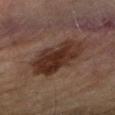Part of a total-body skin-imaging series; this lesion was reviewed on a skin check and was not flagged for biopsy.
Captured under cross-polarized illumination.
The lesion's longest dimension is about 6.5 mm.
A close-up tile cropped from a whole-body skin photograph, about 15 mm across.
A female subject aged around 60.
From the right upper arm.